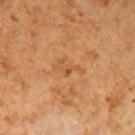The lesion was tiled from a total-body skin photograph and was not biopsied.
The tile uses cross-polarized illumination.
A male patient in their 60s.
The recorded lesion diameter is about 3 mm.
The total-body-photography lesion software estimated a lesion area of about 3.5 mm², a shape eccentricity near 0.85, and a symmetry-axis asymmetry near 0.55. And it measured a border-irregularity rating of about 6.5/10, a color-variation rating of about 1.5/10, and radial color variation of about 0.5. The software also gave an automated nevus-likeness rating near 0 out of 100 and a lesion-detection confidence of about 100/100.
A lesion tile, about 15 mm wide, cut from a 3D total-body photograph.
On the left upper arm.